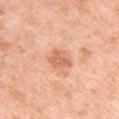This lesion was catalogued during total-body skin photography and was not selected for biopsy. A roughly 15 mm field-of-view crop from a total-body skin photograph. A female patient about 40 years old. Measured at roughly 3 mm in maximum diameter. The lesion is on the arm. Automated tile analysis of the lesion measured a footprint of about 6 mm², a shape eccentricity near 0.65, and a symmetry-axis asymmetry near 0.3. It also reported a mean CIELAB color near L≈66 a*≈26 b*≈36, about 10 CIELAB-L* units darker than the surrounding skin, and a lesion-to-skin contrast of about 6.5 (normalized; higher = more distinct). The analysis additionally found a nevus-likeness score of about 0/100.